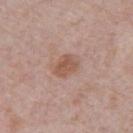Clinical impression:
The lesion was photographed on a routine skin check and not biopsied; there is no pathology result.
Image and clinical context:
A male subject, aged 68–72. A region of skin cropped from a whole-body photographic capture, roughly 15 mm wide. Located on the front of the torso.